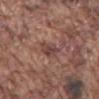follow-up=catalogued during a skin exam; not biopsied | body site=the mid back | image source=15 mm crop, total-body photography | illumination=white-light illumination | subject=male, about 75 years old | size=≈3 mm.